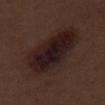Q: Was this lesion biopsied?
A: total-body-photography surveillance lesion; no biopsy
Q: How was this image acquired?
A: 15 mm crop, total-body photography
Q: Who is the patient?
A: male, aged around 70
Q: What did automated image analysis measure?
A: an outline eccentricity of about 0.9 (0 = round, 1 = elongated) and two-axis asymmetry of about 0.25; roughly 8 lightness units darker than nearby skin and a normalized lesion–skin contrast near 11
Q: Where on the body is the lesion?
A: the right thigh
Q: How was the tile lit?
A: white-light
Q: What is the lesion's diameter?
A: ≈10.5 mm